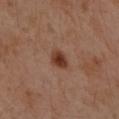workup — no biopsy performed (imaged during a skin exam); subject — female, approximately 40 years of age; lesion size — about 3 mm; image source — ~15 mm tile from a whole-body skin photo; location — the left forearm; illumination — cross-polarized.